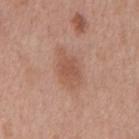tile lighting=white-light; image source=15 mm crop, total-body photography; site=the mid back; subject=male, in their 70s.